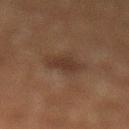<tbp_lesion>
  <biopsy_status>not biopsied; imaged during a skin examination</biopsy_status>
  <site>left lower leg</site>
  <lesion_size>
    <long_diameter_mm_approx>3.0</long_diameter_mm_approx>
  </lesion_size>
  <patient>
    <sex>male</sex>
    <age_approx>65</age_approx>
  </patient>
  <lighting>cross-polarized</lighting>
  <image>
    <source>total-body photography crop</source>
    <field_of_view_mm>15</field_of_view_mm>
  </image>
</tbp_lesion>